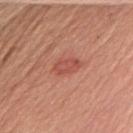biopsy status: imaged on a skin check; not biopsied | site: the left upper arm | TBP lesion metrics: an automated nevus-likeness rating near 55 out of 100 and a lesion-detection confidence of about 100/100 | acquisition: total-body-photography crop, ~15 mm field of view | subject: female, roughly 55 years of age.